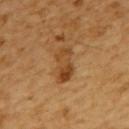Part of a total-body skin-imaging series; this lesion was reviewed on a skin check and was not flagged for biopsy. A 15 mm close-up extracted from a 3D total-body photography capture. The lesion is located on the upper back. An algorithmic analysis of the crop reported an average lesion color of about L≈46 a*≈22 b*≈40 (CIELAB), a lesion–skin lightness drop of about 10, and a normalized lesion–skin contrast near 7.5. The tile uses cross-polarized illumination. A female subject aged around 55. Measured at roughly 4.5 mm in maximum diameter.